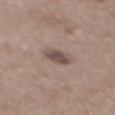Image and clinical context:
The recorded lesion diameter is about 3 mm. A close-up tile cropped from a whole-body skin photograph, about 15 mm across. Automated tile analysis of the lesion measured a border-irregularity rating of about 2.5/10, a color-variation rating of about 2.5/10, and radial color variation of about 1. The patient is a male aged approximately 50. Captured under white-light illumination. The lesion is located on the mid back.